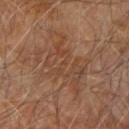{"biopsy_status": "not biopsied; imaged during a skin examination"}